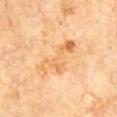Q: Was a biopsy performed?
A: no biopsy performed (imaged during a skin exam)
Q: What are the patient's age and sex?
A: male, in their 70s
Q: Illumination type?
A: cross-polarized
Q: How large is the lesion?
A: about 6.5 mm
Q: What kind of image is this?
A: ~15 mm tile from a whole-body skin photo
Q: What is the anatomic site?
A: the left upper arm
Q: What did automated image analysis measure?
A: a lesion color around L≈70 a*≈22 b*≈44 in CIELAB and a lesion-to-skin contrast of about 6 (normalized; higher = more distinct); a border-irregularity index near 8/10, a within-lesion color-variation index near 4.5/10, and peripheral color asymmetry of about 1